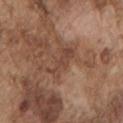A male subject in their mid-70s.
Cropped from a total-body skin-imaging series; the visible field is about 15 mm.
On the arm.
The total-body-photography lesion software estimated a lesion color around L≈44 a*≈19 b*≈27 in CIELAB and about 7 CIELAB-L* units darker than the surrounding skin. The software also gave a nevus-likeness score of about 0/100.
Approximately 4 mm at its widest.
This is a white-light tile.